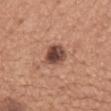biopsy status = no biopsy performed (imaged during a skin exam); tile lighting = white-light illumination; subject = female, about 55 years old; site = the back; acquisition = ~15 mm crop, total-body skin-cancer survey; diameter = ~3.5 mm (longest diameter).